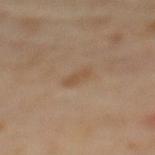Clinical impression:
This lesion was catalogued during total-body skin photography and was not selected for biopsy.
Background:
Automated tile analysis of the lesion measured an area of roughly 3 mm², an outline eccentricity of about 0.9 (0 = round, 1 = elongated), and two-axis asymmetry of about 0.2. The software also gave border irregularity of about 2.5 on a 0–10 scale, internal color variation of about 0 on a 0–10 scale, and peripheral color asymmetry of about 0. A female patient in their 60s. This is a cross-polarized tile. From the upper back. Longest diameter approximately 3 mm. A roughly 15 mm field-of-view crop from a total-body skin photograph.